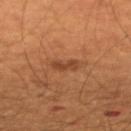{
  "biopsy_status": "not biopsied; imaged during a skin examination",
  "site": "right upper arm",
  "lighting": "cross-polarized",
  "patient": {
    "sex": "male",
    "age_approx": 70
  },
  "image": {
    "source": "total-body photography crop",
    "field_of_view_mm": 15
  },
  "lesion_size": {
    "long_diameter_mm_approx": 2.5
  },
  "automated_metrics": {
    "cielab_L": 42,
    "cielab_a": 23,
    "cielab_b": 34,
    "vs_skin_darker_L": 8.0,
    "vs_skin_contrast_norm": 6.5,
    "nevus_likeness_0_100": 10
  }
}